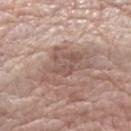{
  "biopsy_status": "not biopsied; imaged during a skin examination",
  "automated_metrics": {
    "eccentricity": 0.8,
    "shape_asymmetry": 0.45,
    "border_irregularity_0_10": 7.0
  },
  "site": "left forearm",
  "lesion_size": {
    "long_diameter_mm_approx": 6.0
  },
  "lighting": "white-light",
  "image": {
    "source": "total-body photography crop",
    "field_of_view_mm": 15
  },
  "patient": {
    "sex": "female",
    "age_approx": 70
  }
}